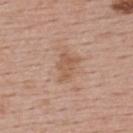biopsy status — catalogued during a skin exam; not biopsied | patient — female, in their mid-50s | diameter — ~3.5 mm (longest diameter) | automated metrics — a mean CIELAB color near L≈56 a*≈20 b*≈31 and roughly 8 lightness units darker than nearby skin; a border-irregularity rating of about 4/10, a within-lesion color-variation index near 2.5/10, and radial color variation of about 1 | acquisition — ~15 mm tile from a whole-body skin photo | site — the upper back | lighting — white-light illumination.